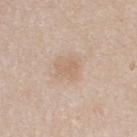{
  "biopsy_status": "not biopsied; imaged during a skin examination",
  "patient": {
    "sex": "male",
    "age_approx": 35
  },
  "automated_metrics": {
    "area_mm2_approx": 4.0,
    "eccentricity": 0.65,
    "shape_asymmetry": 0.3,
    "cielab_L": 64,
    "cielab_a": 16,
    "cielab_b": 30,
    "vs_skin_darker_L": 6.0,
    "vs_skin_contrast_norm": 5.0
  },
  "lighting": "white-light",
  "image": {
    "source": "total-body photography crop",
    "field_of_view_mm": 15
  },
  "site": "back",
  "lesion_size": {
    "long_diameter_mm_approx": 2.5
  }
}